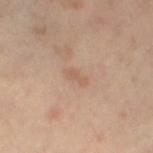notes = no biopsy performed (imaged during a skin exam); patient = female, aged 48–52; imaging modality = 15 mm crop, total-body photography; lesion diameter = ≈3 mm; illumination = cross-polarized; location = the left thigh.